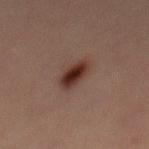workup: catalogued during a skin exam; not biopsied | diameter: ~4 mm (longest diameter) | patient: male, aged around 30 | acquisition: 15 mm crop, total-body photography | location: the mid back | image-analysis metrics: a mean CIELAB color near L≈25 a*≈17 b*≈20, a lesion–skin lightness drop of about 12, and a lesion-to-skin contrast of about 12 (normalized; higher = more distinct); an automated nevus-likeness rating near 100 out of 100 and a lesion-detection confidence of about 100/100.